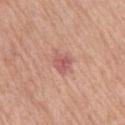Impression:
Imaged during a routine full-body skin examination; the lesion was not biopsied and no histopathology is available.
Image and clinical context:
Cropped from a whole-body photographic skin survey; the tile spans about 15 mm. A male patient, about 60 years old. Imaged with white-light lighting. Located on the arm. The lesion's longest dimension is about 3 mm.